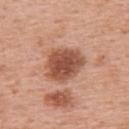Assessment:
The lesion was tiled from a total-body skin photograph and was not biopsied.
Background:
The recorded lesion diameter is about 5 mm. The lesion is located on the back. A female subject in their 50s. An algorithmic analysis of the crop reported a lesion–skin lightness drop of about 15 and a lesion-to-skin contrast of about 10 (normalized; higher = more distinct). It also reported a border-irregularity rating of about 2/10, internal color variation of about 3.5 on a 0–10 scale, and peripheral color asymmetry of about 1. And it measured a nevus-likeness score of about 80/100. This is a white-light tile. A lesion tile, about 15 mm wide, cut from a 3D total-body photograph.